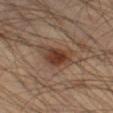{
  "biopsy_status": "not biopsied; imaged during a skin examination",
  "lighting": "cross-polarized",
  "image": {
    "source": "total-body photography crop",
    "field_of_view_mm": 15
  },
  "patient": {
    "sex": "male",
    "age_approx": 45
  },
  "automated_metrics": {
    "area_mm2_approx": 9.0,
    "eccentricity": 0.75,
    "shape_asymmetry": 0.3,
    "nevus_likeness_0_100": 95,
    "lesion_detection_confidence_0_100": 100
  },
  "site": "left thigh",
  "lesion_size": {
    "long_diameter_mm_approx": 4.5
  }
}